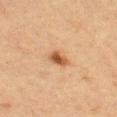| field | value |
|---|---|
| patient | female, approximately 55 years of age |
| imaging modality | ~15 mm tile from a whole-body skin photo |
| body site | the left thigh |